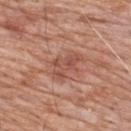- workup — no biopsy performed (imaged during a skin exam)
- site — the upper back
- patient — male, aged around 80
- image — ~15 mm crop, total-body skin-cancer survey
- illumination — white-light